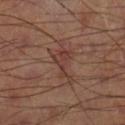biopsy status — imaged on a skin check; not biopsied | TBP lesion metrics — a lesion area of about 5 mm² and a shape-asymmetry score of about 0.7 (0 = symmetric); a lesion color around L≈39 a*≈21 b*≈24 in CIELAB; a border-irregularity index near 8.5/10, a within-lesion color-variation index near 2.5/10, and a peripheral color-asymmetry measure near 1 | body site — the right lower leg | image — total-body-photography crop, ~15 mm field of view.